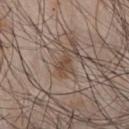<tbp_lesion>
<biopsy_status>not biopsied; imaged during a skin examination</biopsy_status>
<image>
  <source>total-body photography crop</source>
  <field_of_view_mm>15</field_of_view_mm>
</image>
<lighting>white-light</lighting>
<patient>
  <sex>male</sex>
  <age_approx>45</age_approx>
</patient>
<site>chest</site>
</tbp_lesion>